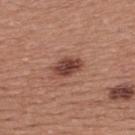Clinical impression: The lesion was tiled from a total-body skin photograph and was not biopsied. Clinical summary: Automated tile analysis of the lesion measured a lesion area of about 6.5 mm² and a shape-asymmetry score of about 0.2 (0 = symmetric). It also reported a mean CIELAB color near L≈43 a*≈24 b*≈27 and a lesion–skin lightness drop of about 13. Approximately 3.5 mm at its widest. A male subject roughly 40 years of age. Located on the upper back. A close-up tile cropped from a whole-body skin photograph, about 15 mm across. Imaged with white-light lighting.